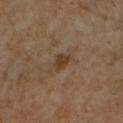Captured during whole-body skin photography for melanoma surveillance; the lesion was not biopsied.
Located on the right upper arm.
Approximately 2.5 mm at its widest.
Automated image analysis of the tile measured a lesion area of about 3.5 mm², an outline eccentricity of about 0.7 (0 = round, 1 = elongated), and two-axis asymmetry of about 0.3. And it measured a mean CIELAB color near L≈39 a*≈16 b*≈29 and a lesion-to-skin contrast of about 7.5 (normalized; higher = more distinct). It also reported border irregularity of about 2.5 on a 0–10 scale, a within-lesion color-variation index near 1.5/10, and a peripheral color-asymmetry measure near 0.5.
Imaged with cross-polarized lighting.
The subject is a female aged approximately 55.
A 15 mm close-up tile from a total-body photography series done for melanoma screening.